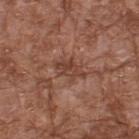<record>
<biopsy_status>not biopsied; imaged during a skin examination</biopsy_status>
<lighting>white-light</lighting>
<image>
  <source>total-body photography crop</source>
  <field_of_view_mm>15</field_of_view_mm>
</image>
<site>upper back</site>
<patient>
  <sex>male</sex>
  <age_approx>75</age_approx>
</patient>
</record>